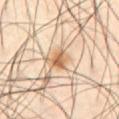workup = imaged on a skin check; not biopsied.